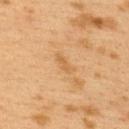Notes:
- follow-up · no biopsy performed (imaged during a skin exam)
- body site · the upper back
- image source · total-body-photography crop, ~15 mm field of view
- subject · female, roughly 40 years of age
- illumination · cross-polarized
- diameter · about 3 mm